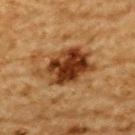Impression:
The lesion was tiled from a total-body skin photograph and was not biopsied.
Background:
A male patient, aged approximately 85 or older. Located on the upper back. A 15 mm close-up tile from a total-body photography series done for melanoma screening.